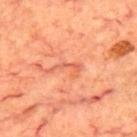Q: Was this lesion biopsied?
A: imaged on a skin check; not biopsied
Q: What kind of image is this?
A: 15 mm crop, total-body photography
Q: Where on the body is the lesion?
A: the upper back
Q: Illumination type?
A: cross-polarized illumination
Q: Who is the patient?
A: male, approximately 70 years of age
Q: Automated lesion metrics?
A: a footprint of about 3.5 mm², an eccentricity of roughly 0.9, and a shape-asymmetry score of about 0.65 (0 = symmetric); an average lesion color of about L≈61 a*≈33 b*≈37 (CIELAB) and a lesion–skin lightness drop of about 8; a nevus-likeness score of about 0/100 and a lesion-detection confidence of about 95/100
Q: Lesion size?
A: about 3.5 mm